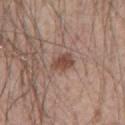Recorded during total-body skin imaging; not selected for excision or biopsy.
From the chest.
This is a white-light tile.
Longest diameter approximately 3 mm.
A male subject aged approximately 55.
The lesion-visualizer software estimated a classifier nevus-likeness of about 85/100 and a lesion-detection confidence of about 100/100.
Cropped from a whole-body photographic skin survey; the tile spans about 15 mm.